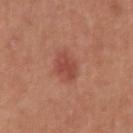{"biopsy_status": "not biopsied; imaged during a skin examination", "image": {"source": "total-body photography crop", "field_of_view_mm": 15}, "lesion_size": {"long_diameter_mm_approx": 3.0}, "patient": {"sex": "male", "age_approx": 55}, "site": "right upper arm"}